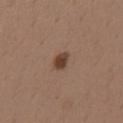Assessment: The lesion was photographed on a routine skin check and not biopsied; there is no pathology result. Background: Automated image analysis of the tile measured a normalized border contrast of about 10. And it measured border irregularity of about 1.5 on a 0–10 scale, internal color variation of about 2.5 on a 0–10 scale, and a peripheral color-asymmetry measure near 1. A male subject roughly 40 years of age. On the mid back. A 15 mm close-up extracted from a 3D total-body photography capture. Measured at roughly 2.5 mm in maximum diameter.